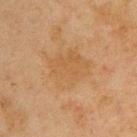biopsy status: catalogued during a skin exam; not biopsied | illumination: cross-polarized | location: the upper back | automated lesion analysis: a symmetry-axis asymmetry near 0.25; a mean CIELAB color near L≈46 a*≈16 b*≈33, about 4 CIELAB-L* units darker than the surrounding skin, and a normalized lesion–skin contrast near 4.5; an automated nevus-likeness rating near 0 out of 100 | size: ≈5 mm | subject: male, about 45 years old | image source: ~15 mm tile from a whole-body skin photo.